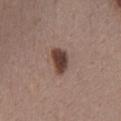Clinical impression:
Imaged during a routine full-body skin examination; the lesion was not biopsied and no histopathology is available.
Background:
An algorithmic analysis of the crop reported an area of roughly 5.5 mm², a shape eccentricity near 0.85, and a symmetry-axis asymmetry near 0.35. And it measured a lesion color around L≈40 a*≈18 b*≈22 in CIELAB and about 16 CIELAB-L* units darker than the surrounding skin. And it measured lesion-presence confidence of about 100/100. The lesion is on the chest. About 3.5 mm across. A close-up tile cropped from a whole-body skin photograph, about 15 mm across. A female subject, aged 48–52.